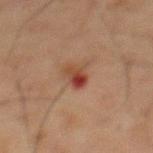{
  "biopsy_status": "not biopsied; imaged during a skin examination",
  "image": {
    "source": "total-body photography crop",
    "field_of_view_mm": 15
  },
  "lesion_size": {
    "long_diameter_mm_approx": 3.0
  },
  "patient": {
    "sex": "male",
    "age_approx": 75
  },
  "site": "abdomen"
}